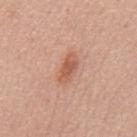| feature | finding |
|---|---|
| lighting | white-light |
| image source | ~15 mm crop, total-body skin-cancer survey |
| lesion size | about 4 mm |
| subject | male, approximately 60 years of age |
| anatomic site | the back |